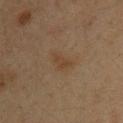Recorded during total-body skin imaging; not selected for excision or biopsy. The lesion-visualizer software estimated a symmetry-axis asymmetry near 0.45. The software also gave a mean CIELAB color near L≈37 a*≈15 b*≈28, about 5 CIELAB-L* units darker than the surrounding skin, and a normalized lesion–skin contrast near 5.5. A 15 mm close-up tile from a total-body photography series done for melanoma screening. Captured under cross-polarized illumination. From the front of the torso. About 3 mm across. A male subject roughly 35 years of age.